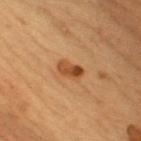Assessment: Captured during whole-body skin photography for melanoma surveillance; the lesion was not biopsied. Context: The lesion-visualizer software estimated an area of roughly 4.5 mm² and an eccentricity of roughly 0.8. And it measured border irregularity of about 2.5 on a 0–10 scale, a within-lesion color-variation index near 6/10, and radial color variation of about 2. Imaged with cross-polarized lighting. A roughly 15 mm field-of-view crop from a total-body skin photograph. A male subject aged approximately 50. The lesion's longest dimension is about 3 mm. The lesion is on the chest.